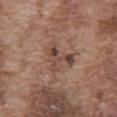Automated image analysis of the tile measured a lesion area of about 6.5 mm², an eccentricity of roughly 0.7, and two-axis asymmetry of about 0.65. And it measured a lesion color around L≈44 a*≈19 b*≈24 in CIELAB. The software also gave an automated nevus-likeness rating near 30 out of 100.
Cropped from a whole-body photographic skin survey; the tile spans about 15 mm.
Longest diameter approximately 4 mm.
This is a white-light tile.
A male subject, roughly 75 years of age.
On the abdomen.